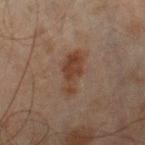Background: A region of skin cropped from a whole-body photographic capture, roughly 15 mm wide. Longest diameter approximately 5 mm. The patient is a male aged 43 to 47. Located on the leg. The total-body-photography lesion software estimated a mean CIELAB color near L≈32 a*≈15 b*≈23, roughly 7 lightness units darker than nearby skin, and a normalized border contrast of about 7.5. The software also gave a color-variation rating of about 4/10.